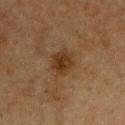The total-body-photography lesion software estimated a lesion color around L≈27 a*≈16 b*≈27 in CIELAB, roughly 8 lightness units darker than nearby skin, and a normalized border contrast of about 9. It also reported a classifier nevus-likeness of about 75/100. A male patient approximately 75 years of age. Imaged with cross-polarized lighting. Longest diameter approximately 3 mm. The lesion is located on the chest. A 15 mm crop from a total-body photograph taken for skin-cancer surveillance.